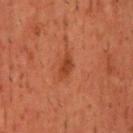Clinical impression:
Part of a total-body skin-imaging series; this lesion was reviewed on a skin check and was not flagged for biopsy.
Acquisition and patient details:
Captured under cross-polarized illumination. An algorithmic analysis of the crop reported a lesion color around L≈39 a*≈28 b*≈35 in CIELAB and a lesion-to-skin contrast of about 7 (normalized; higher = more distinct). The software also gave a border-irregularity rating of about 3/10 and a within-lesion color-variation index near 1/10. It also reported an automated nevus-likeness rating near 65 out of 100 and a lesion-detection confidence of about 100/100. Located on the head or neck. Cropped from a whole-body photographic skin survey; the tile spans about 15 mm. The subject is a male about 50 years old.